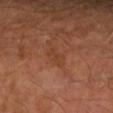Recorded during total-body skin imaging; not selected for excision or biopsy. This is a cross-polarized tile. An algorithmic analysis of the crop reported about 5 CIELAB-L* units darker than the surrounding skin and a lesion-to-skin contrast of about 4.5 (normalized; higher = more distinct). A 15 mm close-up tile from a total-body photography series done for melanoma screening. A male subject approximately 55 years of age. Located on the right forearm.